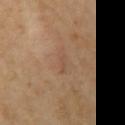Clinical impression:
Imaged during a routine full-body skin examination; the lesion was not biopsied and no histopathology is available.
Acquisition and patient details:
The total-body-photography lesion software estimated a mean CIELAB color near L≈51 a*≈18 b*≈31. The software also gave a border-irregularity index near 3.5/10 and a within-lesion color-variation index near 0/10. This is a cross-polarized tile. A female subject aged around 65. About 2.5 mm across. A close-up tile cropped from a whole-body skin photograph, about 15 mm across. The lesion is on the left upper arm.